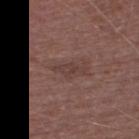Q: Was a biopsy performed?
A: imaged on a skin check; not biopsied
Q: What lighting was used for the tile?
A: white-light
Q: What is the lesion's diameter?
A: ~3.5 mm (longest diameter)
Q: What kind of image is this?
A: 15 mm crop, total-body photography
Q: Who is the patient?
A: male, aged 73 to 77
Q: What is the anatomic site?
A: the left thigh
Q: What did automated image analysis measure?
A: an average lesion color of about L≈39 a*≈18 b*≈21 (CIELAB), a lesion–skin lightness drop of about 6, and a normalized lesion–skin contrast near 5.5; a nevus-likeness score of about 0/100